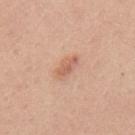Notes:
– notes · imaged on a skin check; not biopsied
– subject · male, aged approximately 30
– site · the mid back
– image · ~15 mm tile from a whole-body skin photo